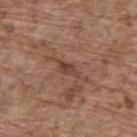Findings:
- biopsy status: catalogued during a skin exam; not biopsied
- illumination: white-light
- body site: the upper back
- patient: male, roughly 70 years of age
- lesion size: about 3 mm
- image source: ~15 mm tile from a whole-body skin photo
- automated lesion analysis: a mean CIELAB color near L≈41 a*≈21 b*≈25, a lesion–skin lightness drop of about 9, and a normalized lesion–skin contrast near 7.5; a nevus-likeness score of about 0/100 and a lesion-detection confidence of about 85/100